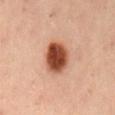Clinical impression:
This lesion was catalogued during total-body skin photography and was not selected for biopsy.
Acquisition and patient details:
On the back. This is a cross-polarized tile. A male patient in their mid-50s. A 15 mm crop from a total-body photograph taken for skin-cancer surveillance. An algorithmic analysis of the crop reported a mean CIELAB color near L≈47 a*≈27 b*≈33 and a lesion–skin lightness drop of about 20. And it measured a border-irregularity rating of about 1.5/10 and a within-lesion color-variation index near 5.5/10.